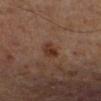– follow-up — catalogued during a skin exam; not biopsied
– lesion size — about 3 mm
– image source — ~15 mm tile from a whole-body skin photo
– patient — male, about 65 years old
– body site — the left lower leg